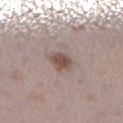- notes — catalogued during a skin exam; not biopsied
- body site — the right lower leg
- subject — male, approximately 40 years of age
- image source — 15 mm crop, total-body photography